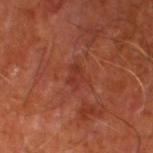* workup · total-body-photography surveillance lesion; no biopsy
* patient · male, roughly 65 years of age
* body site · the left thigh
* illumination · cross-polarized
* image source · 15 mm crop, total-body photography
* diameter · ≈2.5 mm